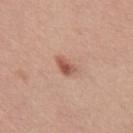Assessment:
The lesion was photographed on a routine skin check and not biopsied; there is no pathology result.
Context:
From the upper back. The subject is a male aged 38 to 42. The total-body-photography lesion software estimated a color-variation rating of about 3.5/10. The software also gave a lesion-detection confidence of about 100/100. This image is a 15 mm lesion crop taken from a total-body photograph. Approximately 2.5 mm at its widest. This is a white-light tile.